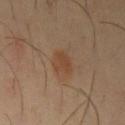Recorded during total-body skin imaging; not selected for excision or biopsy. A male subject, in their 40s. On the left upper arm. Cropped from a whole-body photographic skin survey; the tile spans about 15 mm. About 2.5 mm across. The tile uses cross-polarized illumination. The total-body-photography lesion software estimated an area of roughly 5.5 mm², an outline eccentricity of about 0.25 (0 = round, 1 = elongated), and a symmetry-axis asymmetry near 0.3. It also reported border irregularity of about 2.5 on a 0–10 scale, a color-variation rating of about 1.5/10, and peripheral color asymmetry of about 0.5.